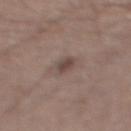biopsy status = no biopsy performed (imaged during a skin exam) | body site = the right thigh | patient = male, roughly 65 years of age | lesion diameter = about 2.5 mm | tile lighting = white-light illumination | image-analysis metrics = an average lesion color of about L≈44 a*≈15 b*≈19 (CIELAB) and roughly 10 lightness units darker than nearby skin; a classifier nevus-likeness of about 10/100 | acquisition = 15 mm crop, total-body photography.